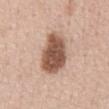Clinical impression: Recorded during total-body skin imaging; not selected for excision or biopsy. Background: An algorithmic analysis of the crop reported a lesion area of about 16 mm², a shape eccentricity near 0.75, and two-axis asymmetry of about 0.15. And it measured an average lesion color of about L≈53 a*≈21 b*≈28 (CIELAB). The software also gave a nevus-likeness score of about 95/100 and a detector confidence of about 100 out of 100 that the crop contains a lesion. Approximately 6 mm at its widest. A male patient in their 50s. The lesion is located on the abdomen. A close-up tile cropped from a whole-body skin photograph, about 15 mm across. Captured under white-light illumination.